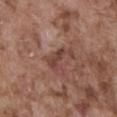Assessment:
This lesion was catalogued during total-body skin photography and was not selected for biopsy.
Image and clinical context:
The total-body-photography lesion software estimated a lesion area of about 5.5 mm², a shape eccentricity near 0.85, and two-axis asymmetry of about 0.55. The analysis additionally found a lesion color around L≈42 a*≈21 b*≈24 in CIELAB, a lesion–skin lightness drop of about 8, and a normalized lesion–skin contrast near 6.5. Located on the abdomen. About 3.5 mm across. A male subject aged 73–77. Cropped from a whole-body photographic skin survey; the tile spans about 15 mm.